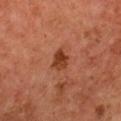Captured during whole-body skin photography for melanoma surveillance; the lesion was not biopsied. A region of skin cropped from a whole-body photographic capture, roughly 15 mm wide. On the chest. Imaged with cross-polarized lighting. A female subject roughly 60 years of age. About 3 mm across.